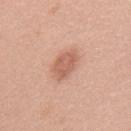| key | value |
|---|---|
| image | 15 mm crop, total-body photography |
| site | the back |
| tile lighting | white-light illumination |
| patient | female, about 40 years old |